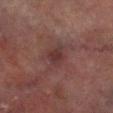Recorded during total-body skin imaging; not selected for excision or biopsy. Captured under cross-polarized illumination. A 15 mm close-up tile from a total-body photography series done for melanoma screening. From the right lower leg. A male subject aged around 75. An algorithmic analysis of the crop reported a lesion area of about 4.5 mm², an outline eccentricity of about 0.6 (0 = round, 1 = elongated), and a symmetry-axis asymmetry near 0.25. It also reported a lesion color around L≈27 a*≈18 b*≈16 in CIELAB, a lesion–skin lightness drop of about 6, and a normalized lesion–skin contrast near 6.5. The analysis additionally found a border-irregularity rating of about 2.5/10 and a within-lesion color-variation index near 2/10. And it measured a nevus-likeness score of about 5/100. Measured at roughly 2.5 mm in maximum diameter.